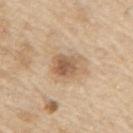Q: Was a biopsy performed?
A: imaged on a skin check; not biopsied
Q: Lesion location?
A: the right upper arm
Q: How was this image acquired?
A: ~15 mm tile from a whole-body skin photo
Q: Patient demographics?
A: male, approximately 70 years of age
Q: Illumination type?
A: white-light illumination